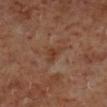Impression: The lesion was photographed on a routine skin check and not biopsied; there is no pathology result. Background: The total-body-photography lesion software estimated an average lesion color of about L≈35 a*≈20 b*≈27 (CIELAB), a lesion–skin lightness drop of about 6, and a normalized lesion–skin contrast near 6. The software also gave a classifier nevus-likeness of about 0/100 and a detector confidence of about 100 out of 100 that the crop contains a lesion. A male subject in their 60s. From the right lower leg. A region of skin cropped from a whole-body photographic capture, roughly 15 mm wide.